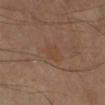Case summary:
– workup: no biopsy performed (imaged during a skin exam)
– subject: female, about 60 years old
– location: the left leg
– image: 15 mm crop, total-body photography
– lesion size: ~2.5 mm (longest diameter)
– illumination: cross-polarized illumination